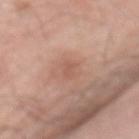The lesion was photographed on a routine skin check and not biopsied; there is no pathology result.
A lesion tile, about 15 mm wide, cut from a 3D total-body photograph.
An algorithmic analysis of the crop reported a classifier nevus-likeness of about 0/100 and a lesion-detection confidence of about 100/100.
This is a white-light tile.
The lesion's longest dimension is about 2.5 mm.
A male subject approximately 70 years of age.
The lesion is on the mid back.